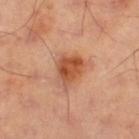No biopsy was performed on this lesion — it was imaged during a full skin examination and was not determined to be concerning. On the left thigh. Imaged with cross-polarized lighting. A 15 mm crop from a total-body photograph taken for skin-cancer surveillance. Measured at roughly 4 mm in maximum diameter. The lesion-visualizer software estimated a footprint of about 9 mm², a shape eccentricity near 0.6, and two-axis asymmetry of about 0.25. And it measured a lesion–skin lightness drop of about 12 and a lesion-to-skin contrast of about 9 (normalized; higher = more distinct). It also reported a color-variation rating of about 5/10 and peripheral color asymmetry of about 2. The software also gave a detector confidence of about 100 out of 100 that the crop contains a lesion.